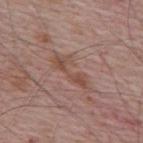biopsy status = imaged on a skin check; not biopsied
acquisition = 15 mm crop, total-body photography
subject = male, roughly 65 years of age
lesion size = about 5.5 mm
body site = the upper back